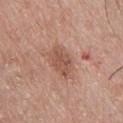| field | value |
|---|---|
| workup | total-body-photography surveillance lesion; no biopsy |
| diameter | about 4 mm |
| image-analysis metrics | an automated nevus-likeness rating near 0 out of 100 |
| subject | male, roughly 45 years of age |
| lighting | white-light |
| imaging modality | 15 mm crop, total-body photography |
| site | the chest |